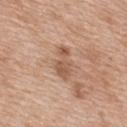No biopsy was performed on this lesion — it was imaged during a full skin examination and was not determined to be concerning. This is a white-light tile. A roughly 15 mm field-of-view crop from a total-body skin photograph. The lesion is located on the upper back. A female patient about 40 years old. The total-body-photography lesion software estimated a border-irregularity rating of about 4/10, a within-lesion color-variation index near 2.5/10, and a peripheral color-asymmetry measure near 1. The software also gave a classifier nevus-likeness of about 0/100 and lesion-presence confidence of about 100/100.